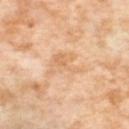No biopsy was performed on this lesion — it was imaged during a full skin examination and was not determined to be concerning. This image is a 15 mm lesion crop taken from a total-body photograph. A female patient, aged 53 to 57. On the left thigh. This is a cross-polarized tile. Approximately 4.5 mm at its widest. Automated tile analysis of the lesion measured a footprint of about 9.5 mm² and an outline eccentricity of about 0.55 (0 = round, 1 = elongated).